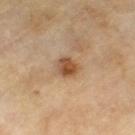– acquisition: total-body-photography crop, ~15 mm field of view
– image-analysis metrics: a border-irregularity index near 2.5/10 and peripheral color asymmetry of about 1.5
– lighting: cross-polarized
– subject: male, aged around 65